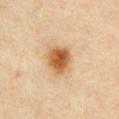The lesion was photographed on a routine skin check and not biopsied; there is no pathology result. Automated image analysis of the tile measured a normalized border contrast of about 9.5. A 15 mm close-up extracted from a 3D total-body photography capture. From the chest. The lesion's longest dimension is about 4 mm. Imaged with cross-polarized lighting. A male subject, aged 38 to 42.